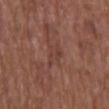Captured during whole-body skin photography for melanoma surveillance; the lesion was not biopsied.